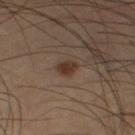Located on the leg.
The patient is a male in their mid-60s.
A roughly 15 mm field-of-view crop from a total-body skin photograph.